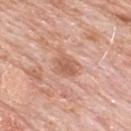biopsy status: imaged on a skin check; not biopsied
image: total-body-photography crop, ~15 mm field of view
automated metrics: about 9 CIELAB-L* units darker than the surrounding skin; a border-irregularity index near 2.5/10 and a within-lesion color-variation index near 3/10; an automated nevus-likeness rating near 0 out of 100 and a lesion-detection confidence of about 100/100
lighting: white-light illumination
anatomic site: the upper back
subject: male, aged approximately 80